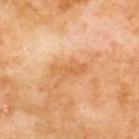workup: imaged on a skin check; not biopsied
automated metrics: a footprint of about 5.5 mm², an outline eccentricity of about 0.9 (0 = round, 1 = elongated), and two-axis asymmetry of about 0.35; an average lesion color of about L≈62 a*≈24 b*≈43 (CIELAB), about 8 CIELAB-L* units darker than the surrounding skin, and a normalized lesion–skin contrast near 6; border irregularity of about 5.5 on a 0–10 scale, a within-lesion color-variation index near 1.5/10, and radial color variation of about 0.5
imaging modality: 15 mm crop, total-body photography
site: the upper back
patient: male, in their 70s
illumination: cross-polarized illumination
size: ~4 mm (longest diameter)